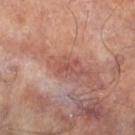Impression: Recorded during total-body skin imaging; not selected for excision or biopsy. Context: This is a cross-polarized tile. The lesion is on the leg. The lesion-visualizer software estimated a mean CIELAB color near L≈49 a*≈26 b*≈25. The analysis additionally found an automated nevus-likeness rating near 0 out of 100 and a lesion-detection confidence of about 100/100. Cropped from a total-body skin-imaging series; the visible field is about 15 mm. About 2.5 mm across. The patient is a male in their mid- to late 60s.